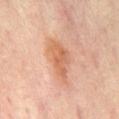workup: imaged on a skin check; not biopsied
patient: male, aged approximately 65
body site: the mid back
automated lesion analysis: a lesion area of about 11 mm² and a shape-asymmetry score of about 0.4 (0 = symmetric); a border-irregularity rating of about 4.5/10, a within-lesion color-variation index near 4/10, and radial color variation of about 1.5; an automated nevus-likeness rating near 20 out of 100 and a detector confidence of about 100 out of 100 that the crop contains a lesion
diameter: ~5.5 mm (longest diameter)
acquisition: total-body-photography crop, ~15 mm field of view
illumination: cross-polarized illumination